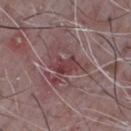| field | value |
|---|---|
| workup | catalogued during a skin exam; not biopsied |